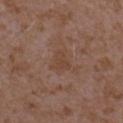Imaged during a routine full-body skin examination; the lesion was not biopsied and no histopathology is available. The tile uses white-light illumination. A female patient, in their mid-30s. Located on the left forearm. Longest diameter approximately 2.5 mm. A roughly 15 mm field-of-view crop from a total-body skin photograph.